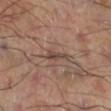Captured during whole-body skin photography for melanoma surveillance; the lesion was not biopsied. A 15 mm close-up extracted from a 3D total-body photography capture. About 3.5 mm across. This is a cross-polarized tile. Located on the right thigh.